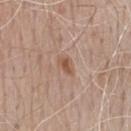• notes · total-body-photography surveillance lesion; no biopsy
• illumination · white-light
• subject · male, aged around 75
• diameter · about 2.5 mm
• body site · the chest
• image source · total-body-photography crop, ~15 mm field of view